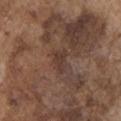  biopsy_status: not biopsied; imaged during a skin examination
  site: left upper arm
  image:
    source: total-body photography crop
    field_of_view_mm: 15
  patient:
    sex: male
    age_approx: 75
  lighting: white-light
  automated_metrics:
    eccentricity: 0.85
    border_irregularity_0_10: 3.5
    color_variation_0_10: 0.5
    peripheral_color_asymmetry: 0.0
    lesion_detection_confidence_0_100: 75